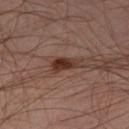biopsy status: total-body-photography surveillance lesion; no biopsy
lesion diameter: about 3 mm
subject: male, aged 43 to 47
automated lesion analysis: an area of roughly 4.5 mm², an eccentricity of roughly 0.85, and a shape-asymmetry score of about 0.35 (0 = symmetric); a lesion color around L≈31 a*≈19 b*≈23 in CIELAB, a lesion–skin lightness drop of about 11, and a normalized lesion–skin contrast near 11; a border-irregularity rating of about 3.5/10 and a within-lesion color-variation index near 4/10; a classifier nevus-likeness of about 95/100 and a detector confidence of about 100 out of 100 that the crop contains a lesion
lighting: cross-polarized illumination
image source: 15 mm crop, total-body photography
body site: the leg